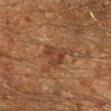workup = no biopsy performed (imaged during a skin exam) | diameter = ~3 mm (longest diameter) | body site = the right lower leg | subject = male, aged around 60 | illumination = cross-polarized illumination | acquisition = 15 mm crop, total-body photography.